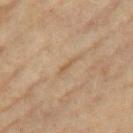| feature | finding |
|---|---|
| notes | total-body-photography surveillance lesion; no biopsy |
| illumination | cross-polarized illumination |
| image-analysis metrics | a border-irregularity index near 4.5/10, a within-lesion color-variation index near 0/10, and radial color variation of about 0; a nevus-likeness score of about 0/100 and lesion-presence confidence of about 50/100 |
| image source | ~15 mm tile from a whole-body skin photo |
| lesion diameter | ≈2.5 mm |
| location | the leg |
| subject | female, aged 73–77 |